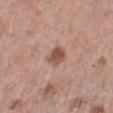Clinical impression:
Imaged during a routine full-body skin examination; the lesion was not biopsied and no histopathology is available.
Image and clinical context:
The lesion is on the right lower leg. Cropped from a total-body skin-imaging series; the visible field is about 15 mm. This is a white-light tile. Longest diameter approximately 2.5 mm. A female subject about 40 years old.